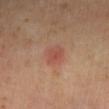{
  "biopsy_status": "not biopsied; imaged during a skin examination",
  "lighting": "cross-polarized",
  "lesion_size": {
    "long_diameter_mm_approx": 3.0
  },
  "automated_metrics": {
    "area_mm2_approx": 5.5,
    "eccentricity": 0.65,
    "shape_asymmetry": 0.2,
    "cielab_L": 49,
    "cielab_a": 25,
    "cielab_b": 28,
    "vs_skin_contrast_norm": 5.0
  },
  "site": "left lower leg",
  "patient": {
    "sex": "male",
    "age_approx": 55
  },
  "image": {
    "source": "total-body photography crop",
    "field_of_view_mm": 15
  }
}